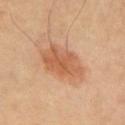Captured during whole-body skin photography for melanoma surveillance; the lesion was not biopsied. Measured at roughly 6 mm in maximum diameter. On the chest. A 15 mm close-up extracted from a 3D total-body photography capture. Automated image analysis of the tile measured a lesion area of about 16 mm², an outline eccentricity of about 0.8 (0 = round, 1 = elongated), and two-axis asymmetry of about 0.15. It also reported a mean CIELAB color near L≈60 a*≈24 b*≈37, about 10 CIELAB-L* units darker than the surrounding skin, and a normalized border contrast of about 7. Imaged with cross-polarized lighting.